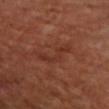Image and clinical context:
This image is a 15 mm lesion crop taken from a total-body photograph. The recorded lesion diameter is about 5 mm. A female patient, aged 48 to 52. On the right upper arm. Captured under cross-polarized illumination.